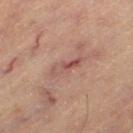Part of a total-body skin-imaging series; this lesion was reviewed on a skin check and was not flagged for biopsy. A close-up tile cropped from a whole-body skin photograph, about 15 mm across. About 4 mm across. A male subject aged 58–62. From the left thigh. Captured under cross-polarized illumination.